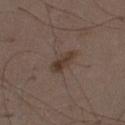Recorded during total-body skin imaging; not selected for excision or biopsy.
A close-up tile cropped from a whole-body skin photograph, about 15 mm across.
The lesion-visualizer software estimated an outline eccentricity of about 0.9 (0 = round, 1 = elongated). The software also gave a lesion color around L≈36 a*≈14 b*≈23 in CIELAB, about 8 CIELAB-L* units darker than the surrounding skin, and a normalized lesion–skin contrast near 8. It also reported a nevus-likeness score of about 30/100 and a lesion-detection confidence of about 100/100.
Located on the abdomen.
Imaged with white-light lighting.
A male patient, roughly 50 years of age.
Longest diameter approximately 3.5 mm.